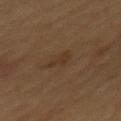Q: Was this lesion biopsied?
A: no biopsy performed (imaged during a skin exam)
Q: Who is the patient?
A: male, aged approximately 60
Q: What kind of image is this?
A: 15 mm crop, total-body photography
Q: Where on the body is the lesion?
A: the mid back